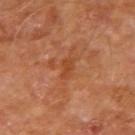biopsy status=no biopsy performed (imaged during a skin exam) | site=the right upper arm | tile lighting=cross-polarized | TBP lesion metrics=an eccentricity of roughly 0.85; a mean CIELAB color near L≈45 a*≈28 b*≈38, a lesion–skin lightness drop of about 6, and a normalized lesion–skin contrast near 5.5 | patient=male, aged 68 to 72 | image source=15 mm crop, total-body photography.